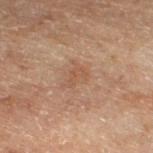Impression: No biopsy was performed on this lesion — it was imaged during a full skin examination and was not determined to be concerning. Image and clinical context: On the left lower leg. Imaged with cross-polarized lighting. Longest diameter approximately 3 mm. The patient is a male aged around 70. Cropped from a whole-body photographic skin survey; the tile spans about 15 mm.